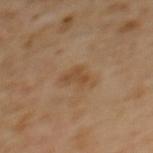biopsy status = imaged on a skin check; not biopsied | location = the upper back | image = ~15 mm tile from a whole-body skin photo | subject = female, in their mid- to late 50s | size = ≈2.5 mm.